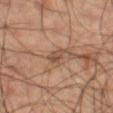{"site": "left thigh", "patient": {"sex": "male", "age_approx": 60}, "image": {"source": "total-body photography crop", "field_of_view_mm": 15}}